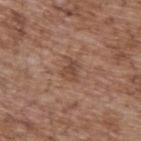{
  "biopsy_status": "not biopsied; imaged during a skin examination",
  "automated_metrics": {
    "nevus_likeness_0_100": 0,
    "lesion_detection_confidence_0_100": 100
  },
  "site": "back",
  "image": {
    "source": "total-body photography crop",
    "field_of_view_mm": 15
  },
  "lighting": "white-light",
  "patient": {
    "sex": "male",
    "age_approx": 70
  },
  "lesion_size": {
    "long_diameter_mm_approx": 2.5
  }
}